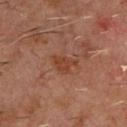<case>
  <biopsy_status>not biopsied; imaged during a skin examination</biopsy_status>
  <patient>
    <sex>male</sex>
    <age_approx>60</age_approx>
  </patient>
  <site>front of the torso</site>
  <image>
    <source>total-body photography crop</source>
    <field_of_view_mm>15</field_of_view_mm>
  </image>
</case>